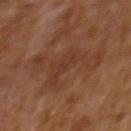| key | value |
|---|---|
| follow-up | total-body-photography surveillance lesion; no biopsy |
| site | the mid back |
| illumination | cross-polarized illumination |
| patient | male, about 65 years old |
| image source | 15 mm crop, total-body photography |
| lesion size | about 7.5 mm |